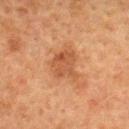This lesion was catalogued during total-body skin photography and was not selected for biopsy. A female subject, aged around 55. The lesion-visualizer software estimated an area of roughly 11 mm² and a shape-asymmetry score of about 0.4 (0 = symmetric). The tile uses cross-polarized illumination. A lesion tile, about 15 mm wide, cut from a 3D total-body photograph. The lesion is located on the back. Approximately 4.5 mm at its widest.